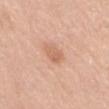Recorded during total-body skin imaging; not selected for excision or biopsy. The recorded lesion diameter is about 3 mm. The subject is a female aged approximately 65. On the abdomen. Captured under white-light illumination. The total-body-photography lesion software estimated a lesion color around L≈63 a*≈23 b*≈33 in CIELAB. And it measured border irregularity of about 2.5 on a 0–10 scale, a within-lesion color-variation index near 2/10, and radial color variation of about 0.5. And it measured a classifier nevus-likeness of about 35/100 and a lesion-detection confidence of about 100/100. This image is a 15 mm lesion crop taken from a total-body photograph.